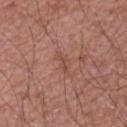This lesion was catalogued during total-body skin photography and was not selected for biopsy.
A 15 mm crop from a total-body photograph taken for skin-cancer surveillance.
An algorithmic analysis of the crop reported a footprint of about 3.5 mm² and an outline eccentricity of about 0.9 (0 = round, 1 = elongated). The analysis additionally found a within-lesion color-variation index near 1/10 and radial color variation of about 0.5.
Captured under white-light illumination.
On the right upper arm.
Measured at roughly 3 mm in maximum diameter.
A male subject aged 48 to 52.